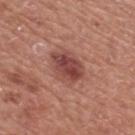image = 15 mm crop, total-body photography
lesion size = about 4.5 mm
patient = male, aged 73 to 77
automated metrics = a nevus-likeness score of about 85/100
site = the mid back